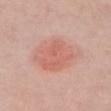Q: Is there a histopathology result?
A: no biopsy performed (imaged during a skin exam)
Q: Who is the patient?
A: female, in their mid- to late 60s
Q: What lighting was used for the tile?
A: white-light illumination
Q: How was this image acquired?
A: ~15 mm tile from a whole-body skin photo
Q: Lesion location?
A: the chest
Q: What did automated image analysis measure?
A: a footprint of about 22 mm² and an eccentricity of roughly 0.65; border irregularity of about 2.5 on a 0–10 scale, a color-variation rating of about 3.5/10, and radial color variation of about 1